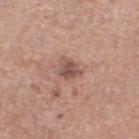Imaged during a routine full-body skin examination; the lesion was not biopsied and no histopathology is available.
The lesion is on the right lower leg.
A male patient aged 58 to 62.
A lesion tile, about 15 mm wide, cut from a 3D total-body photograph.